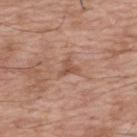follow-up — total-body-photography surveillance lesion; no biopsy
site — the back
lesion size — about 2.5 mm
acquisition — ~15 mm tile from a whole-body skin photo
subject — male, about 70 years old
tile lighting — white-light illumination